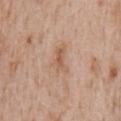  biopsy_status: not biopsied; imaged during a skin examination
  lighting: white-light
  image:
    source: total-body photography crop
    field_of_view_mm: 15
  site: chest
  patient:
    sex: male
    age_approx: 65